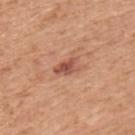Q: Was a biopsy performed?
A: imaged on a skin check; not biopsied